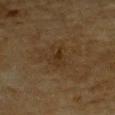No biopsy was performed on this lesion — it was imaged during a full skin examination and was not determined to be concerning. On the chest. A male patient, aged around 85 or older. Captured under cross-polarized illumination. Approximately 3 mm at its widest. Cropped from a total-body skin-imaging series; the visible field is about 15 mm.